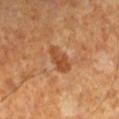workup: total-body-photography surveillance lesion; no biopsy
acquisition: ~15 mm tile from a whole-body skin photo
subject: male, approximately 60 years of age
lighting: cross-polarized illumination
anatomic site: the left lower leg
TBP lesion metrics: a footprint of about 6 mm², a shape eccentricity near 0.8, and two-axis asymmetry of about 0.25; about 9 CIELAB-L* units darker than the surrounding skin and a normalized lesion–skin contrast near 7; internal color variation of about 2.5 on a 0–10 scale and peripheral color asymmetry of about 1; a classifier nevus-likeness of about 25/100 and lesion-presence confidence of about 100/100